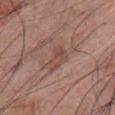Clinical impression:
Imaged during a routine full-body skin examination; the lesion was not biopsied and no histopathology is available.
Acquisition and patient details:
A 15 mm crop from a total-body photograph taken for skin-cancer surveillance. Measured at roughly 4 mm in maximum diameter. The subject is a male aged around 70. The tile uses white-light illumination. Located on the chest.